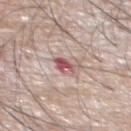Recorded during total-body skin imaging; not selected for excision or biopsy. The patient is a male aged 58–62. The total-body-photography lesion software estimated a lesion area of about 4.5 mm², a shape eccentricity near 0.55, and two-axis asymmetry of about 0.3. The analysis additionally found a border-irregularity index near 3.5/10, internal color variation of about 6 on a 0–10 scale, and radial color variation of about 2. Longest diameter approximately 3 mm. The lesion is located on the chest. A 15 mm crop from a total-body photograph taken for skin-cancer surveillance.